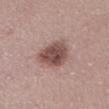Clinical impression: No biopsy was performed on this lesion — it was imaged during a full skin examination and was not determined to be concerning.